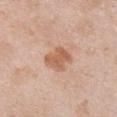Q: Was a biopsy performed?
A: no biopsy performed (imaged during a skin exam)
Q: Where on the body is the lesion?
A: the chest
Q: What are the patient's age and sex?
A: female, in their 50s
Q: Lesion size?
A: ~3 mm (longest diameter)
Q: What kind of image is this?
A: ~15 mm crop, total-body skin-cancer survey